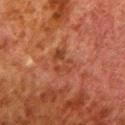{
  "biopsy_status": "not biopsied; imaged during a skin examination",
  "patient": {
    "sex": "male",
    "age_approx": 80
  },
  "site": "left lower leg",
  "lesion_size": {
    "long_diameter_mm_approx": 3.5
  },
  "image": {
    "source": "total-body photography crop",
    "field_of_view_mm": 15
  },
  "automated_metrics": {
    "color_variation_0_10": 4.0,
    "peripheral_color_asymmetry": 1.5
  }
}